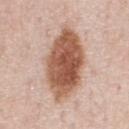  biopsy_status: not biopsied; imaged during a skin examination
  site: chest
  lighting: white-light
  automated_metrics:
    cielab_L: 56
    cielab_a: 22
    cielab_b: 30
    vs_skin_contrast_norm: 11.5
    color_variation_0_10: 6.0
    nevus_likeness_0_100: 100
    lesion_detection_confidence_0_100: 100
  patient:
    sex: male
    age_approx: 60
  image:
    source: total-body photography crop
    field_of_view_mm: 15